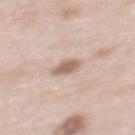Captured during whole-body skin photography for melanoma surveillance; the lesion was not biopsied.
About 2.5 mm across.
Cropped from a total-body skin-imaging series; the visible field is about 15 mm.
The lesion is located on the upper back.
An algorithmic analysis of the crop reported internal color variation of about 1.5 on a 0–10 scale and peripheral color asymmetry of about 0.5.
The subject is a female aged 63 to 67.
Captured under white-light illumination.